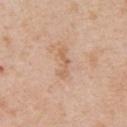Findings:
– biopsy status · imaged on a skin check; not biopsied